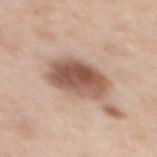The lesion is on the mid back.
A female subject, aged 48 to 52.
The tile uses white-light illumination.
A lesion tile, about 15 mm wide, cut from a 3D total-body photograph.
Automated image analysis of the tile measured a lesion-to-skin contrast of about 10 (normalized; higher = more distinct). The software also gave a within-lesion color-variation index near 7/10 and radial color variation of about 2.5. And it measured an automated nevus-likeness rating near 85 out of 100 and lesion-presence confidence of about 100/100.